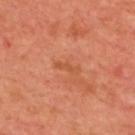| key | value |
|---|---|
| notes | catalogued during a skin exam; not biopsied |
| lesion diameter | ≈3 mm |
| anatomic site | the upper back |
| image source | ~15 mm crop, total-body skin-cancer survey |
| patient | male, roughly 65 years of age |